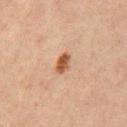No biopsy was performed on this lesion — it was imaged during a full skin examination and was not determined to be concerning.
Longest diameter approximately 2.5 mm.
The lesion is on the mid back.
Imaged with cross-polarized lighting.
A male subject, approximately 65 years of age.
A lesion tile, about 15 mm wide, cut from a 3D total-body photograph.